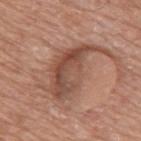The lesion was photographed on a routine skin check and not biopsied; there is no pathology result.
Longest diameter approximately 8.5 mm.
The lesion is located on the back.
A close-up tile cropped from a whole-body skin photograph, about 15 mm across.
The subject is a male in their mid-70s.
Imaged with white-light lighting.